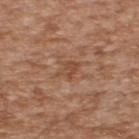| key | value |
|---|---|
| subject | male, approximately 65 years of age |
| image source | ~15 mm tile from a whole-body skin photo |
| TBP lesion metrics | an area of roughly 3.5 mm², an eccentricity of roughly 0.7, and two-axis asymmetry of about 0.35; a lesion–skin lightness drop of about 7 and a normalized border contrast of about 6 |
| lesion size | ~2.5 mm (longest diameter) |
| body site | the upper back |
| lighting | white-light illumination |